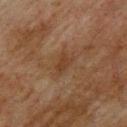The lesion was photographed on a routine skin check and not biopsied; there is no pathology result. A 15 mm close-up tile from a total-body photography series done for melanoma screening. The tile uses cross-polarized illumination. A male patient aged 73–77. The lesion-visualizer software estimated a shape-asymmetry score of about 0.35 (0 = symmetric). The analysis additionally found a mean CIELAB color near L≈32 a*≈17 b*≈26, a lesion–skin lightness drop of about 6, and a lesion-to-skin contrast of about 6 (normalized; higher = more distinct). On the upper back. The recorded lesion diameter is about 2.5 mm.